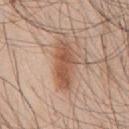The lesion is on the chest. An algorithmic analysis of the crop reported a lesion area of about 12 mm², an eccentricity of roughly 0.9, and two-axis asymmetry of about 0.25. It also reported a border-irregularity index near 3.5/10. It also reported an automated nevus-likeness rating near 85 out of 100 and a lesion-detection confidence of about 100/100. A lesion tile, about 15 mm wide, cut from a 3D total-body photograph. Captured under white-light illumination. A male patient aged approximately 45.